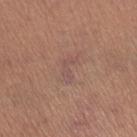{
  "biopsy_status": "not biopsied; imaged during a skin examination",
  "lighting": "white-light",
  "lesion_size": {
    "long_diameter_mm_approx": 3.0
  },
  "automated_metrics": {
    "cielab_L": 51,
    "cielab_a": 20,
    "cielab_b": 22,
    "vs_skin_darker_L": 5.0,
    "vs_skin_contrast_norm": 5.0,
    "color_variation_0_10": 0.0,
    "nevus_likeness_0_100": 0,
    "lesion_detection_confidence_0_100": 95
  },
  "image": {
    "source": "total-body photography crop",
    "field_of_view_mm": 15
  },
  "site": "left thigh",
  "patient": {
    "sex": "female",
    "age_approx": 35
  }
}